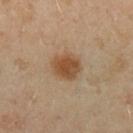body site: the arm
imaging modality: ~15 mm crop, total-body skin-cancer survey
image-analysis metrics: an area of roughly 8 mm², an eccentricity of roughly 0.55, and a shape-asymmetry score of about 0.15 (0 = symmetric); a border-irregularity rating of about 1.5/10, a color-variation rating of about 3/10, and radial color variation of about 1; a classifier nevus-likeness of about 100/100 and a lesion-detection confidence of about 100/100
lighting: cross-polarized
subject: female, approximately 40 years of age
diameter: about 3.5 mm